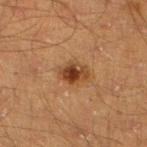The lesion was photographed on a routine skin check and not biopsied; there is no pathology result. Approximately 3.5 mm at its widest. Captured under cross-polarized illumination. A close-up tile cropped from a whole-body skin photograph, about 15 mm across. The subject is a male about 45 years old. Located on the leg.